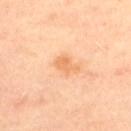Notes:
• biopsy status · imaged on a skin check; not biopsied
• subject · female, approximately 50 years of age
• body site · the chest
• imaging modality · 15 mm crop, total-body photography
• lesion diameter · ≈2.5 mm
• automated metrics · a classifier nevus-likeness of about 35/100 and a lesion-detection confidence of about 100/100
• illumination · cross-polarized